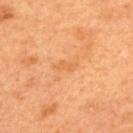- follow-up · total-body-photography surveillance lesion; no biopsy
- imaging modality · ~15 mm tile from a whole-body skin photo
- subject · male, about 50 years old
- diameter · about 2.5 mm
- site · the upper back
- lighting · cross-polarized